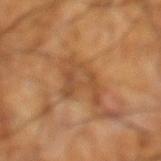No biopsy was performed on this lesion — it was imaged during a full skin examination and was not determined to be concerning.
From the left lower leg.
An algorithmic analysis of the crop reported an area of roughly 11 mm², an eccentricity of roughly 0.85, and a shape-asymmetry score of about 0.55 (0 = symmetric). The software also gave a border-irregularity index near 6.5/10, internal color variation of about 3.5 on a 0–10 scale, and a peripheral color-asymmetry measure near 1.
The tile uses cross-polarized illumination.
A 15 mm close-up tile from a total-body photography series done for melanoma screening.
The lesion's longest dimension is about 5.5 mm.
A male subject in their 60s.